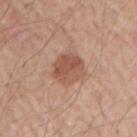The total-body-photography lesion software estimated an area of roughly 10 mm² and a shape-asymmetry score of about 0.15 (0 = symmetric). The analysis additionally found a border-irregularity index near 1.5/10, a color-variation rating of about 4/10, and peripheral color asymmetry of about 1.5. A 15 mm crop from a total-body photograph taken for skin-cancer surveillance. The lesion is located on the right upper arm. A male subject, aged 53 to 57.